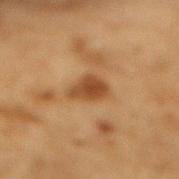The lesion was tiled from a total-body skin photograph and was not biopsied. This is a cross-polarized tile. A roughly 15 mm field-of-view crop from a total-body skin photograph. Approximately 4 mm at its widest. Automated tile analysis of the lesion measured a lesion area of about 7 mm², an eccentricity of roughly 0.8, and a symmetry-axis asymmetry near 0.35. And it measured a border-irregularity rating of about 3/10, internal color variation of about 3 on a 0–10 scale, and peripheral color asymmetry of about 1. And it measured a classifier nevus-likeness of about 100/100 and lesion-presence confidence of about 95/100. The subject is a male aged around 85 or older. On the mid back.